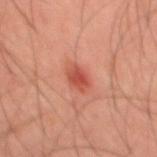notes — total-body-photography surveillance lesion; no biopsy | acquisition — ~15 mm crop, total-body skin-cancer survey | site — the mid back | subject — male, aged around 45.